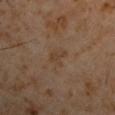image = ~15 mm crop, total-body skin-cancer survey
subject = male, roughly 60 years of age
body site = the left upper arm